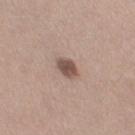notes: no biopsy performed (imaged during a skin exam) | patient: female, about 40 years old | site: the left thigh | image source: ~15 mm crop, total-body skin-cancer survey.